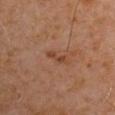  biopsy_status: not biopsied; imaged during a skin examination
  site: right upper arm
  image:
    source: total-body photography crop
    field_of_view_mm: 15
  patient:
    sex: male
    age_approx: 60